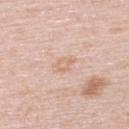Impression: Imaged during a routine full-body skin examination; the lesion was not biopsied and no histopathology is available. Clinical summary: A female subject roughly 65 years of age. A 15 mm close-up extracted from a 3D total-body photography capture. The lesion is located on the upper back.